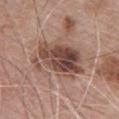- notes: catalogued during a skin exam; not biopsied
- subject: male, aged 63–67
- lesion diameter: ≈7 mm
- body site: the chest
- image source: ~15 mm crop, total-body skin-cancer survey
- illumination: white-light
- automated lesion analysis: an area of roughly 22 mm², an outline eccentricity of about 0.75 (0 = round, 1 = elongated), and a symmetry-axis asymmetry near 0.25; border irregularity of about 4 on a 0–10 scale and radial color variation of about 3